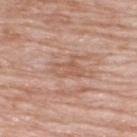The lesion was photographed on a routine skin check and not biopsied; there is no pathology result. The lesion is located on the upper back. An algorithmic analysis of the crop reported a nevus-likeness score of about 0/100 and lesion-presence confidence of about 90/100. A male subject, aged approximately 60. Captured under white-light illumination. The recorded lesion diameter is about 3.5 mm. Cropped from a total-body skin-imaging series; the visible field is about 15 mm.